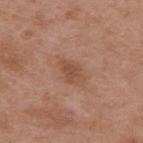Imaged during a routine full-body skin examination; the lesion was not biopsied and no histopathology is available. Approximately 3 mm at its widest. The lesion is on the back. A female patient in their mid- to late 30s. An algorithmic analysis of the crop reported an automated nevus-likeness rating near 5 out of 100 and a lesion-detection confidence of about 100/100. Imaged with white-light lighting. A 15 mm crop from a total-body photograph taken for skin-cancer surveillance.